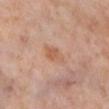* notes: catalogued during a skin exam; not biopsied
* size: ≈3 mm
* location: the left lower leg
* subject: female, aged 53–57
* illumination: cross-polarized
* automated metrics: a border-irregularity index near 3/10, a within-lesion color-variation index near 3/10, and radial color variation of about 1; an automated nevus-likeness rating near 20 out of 100 and a detector confidence of about 100 out of 100 that the crop contains a lesion
* image: ~15 mm crop, total-body skin-cancer survey